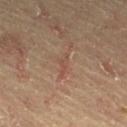Recorded during total-body skin imaging; not selected for excision or biopsy. The tile uses cross-polarized illumination. The lesion is on the right thigh. A roughly 15 mm field-of-view crop from a total-body skin photograph. Measured at roughly 2.5 mm in maximum diameter. The patient is a male roughly 65 years of age.